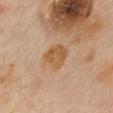| field | value |
|---|---|
| notes | catalogued during a skin exam; not biopsied |
| image source | ~15 mm crop, total-body skin-cancer survey |
| site | the mid back |
| subject | female, roughly 60 years of age |